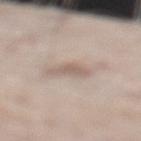Clinical impression:
This lesion was catalogued during total-body skin photography and was not selected for biopsy.
Acquisition and patient details:
This image is a 15 mm lesion crop taken from a total-body photograph. Approximately 3 mm at its widest. Located on the left lower leg. The subject is a male aged approximately 60.